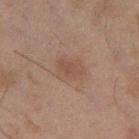- body site · the left thigh
- patient · male, aged around 45
- imaging modality · ~15 mm crop, total-body skin-cancer survey
- lesion diameter · ≈3.5 mm